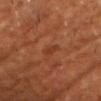| key | value |
|---|---|
| biopsy status | imaged on a skin check; not biopsied |
| acquisition | ~15 mm tile from a whole-body skin photo |
| location | the chest |
| illumination | cross-polarized illumination |
| subject | male, approximately 60 years of age |